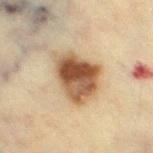Q: Was this lesion biopsied?
A: no biopsy performed (imaged during a skin exam)
Q: What is the lesion's diameter?
A: ≈6 mm
Q: What lighting was used for the tile?
A: cross-polarized
Q: What is the anatomic site?
A: the right thigh
Q: How was this image acquired?
A: 15 mm crop, total-body photography
Q: What are the patient's age and sex?
A: female, aged 53–57